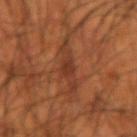A male subject aged 53–57.
From the left forearm.
A 15 mm close-up tile from a total-body photography series done for melanoma screening.
The tile uses cross-polarized illumination.
Automated tile analysis of the lesion measured a footprint of about 9.5 mm², an eccentricity of roughly 0.9, and a symmetry-axis asymmetry near 0.35. It also reported a lesion-detection confidence of about 70/100.